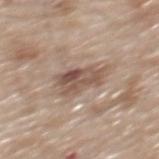Impression:
Recorded during total-body skin imaging; not selected for excision or biopsy.
Context:
Located on the mid back. Automated tile analysis of the lesion measured a footprint of about 10 mm² and an outline eccentricity of about 0.8 (0 = round, 1 = elongated). It also reported a lesion color around L≈53 a*≈17 b*≈25 in CIELAB and a normalized lesion–skin contrast near 8. It also reported a border-irregularity rating of about 4/10, a color-variation rating of about 6.5/10, and a peripheral color-asymmetry measure near 2.5. A male subject, roughly 80 years of age. Captured under white-light illumination. A roughly 15 mm field-of-view crop from a total-body skin photograph.